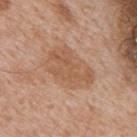<case>
<biopsy_status>not biopsied; imaged during a skin examination</biopsy_status>
<image>
  <source>total-body photography crop</source>
  <field_of_view_mm>15</field_of_view_mm>
</image>
<automated_metrics>
  <border_irregularity_0_10>2.0</border_irregularity_0_10>
  <color_variation_0_10>3.5</color_variation_0_10>
  <peripheral_color_asymmetry>1.0</peripheral_color_asymmetry>
</automated_metrics>
<site>mid back</site>
<lighting>white-light</lighting>
<patient>
  <sex>male</sex>
  <age_approx>65</age_approx>
</patient>
</case>